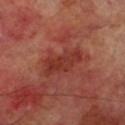notes — no biopsy performed (imaged during a skin exam)
body site — the leg
lesion diameter — ≈5 mm
illumination — cross-polarized
subject — male, aged 68 to 72
imaging modality — ~15 mm tile from a whole-body skin photo